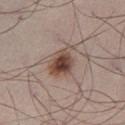Recorded during total-body skin imaging; not selected for excision or biopsy. Cropped from a whole-body photographic skin survey; the tile spans about 15 mm. On the right thigh. The tile uses white-light illumination. A male patient about 50 years old. The lesion's longest dimension is about 4 mm.